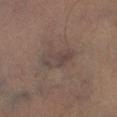follow-up — total-body-photography surveillance lesion; no biopsy | body site — the left leg | patient — male, aged approximately 35 | lighting — cross-polarized | imaging modality — ~15 mm tile from a whole-body skin photo | diameter — ≈4.5 mm.